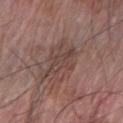<tbp_lesion>
  <biopsy_status>not biopsied; imaged during a skin examination</biopsy_status>
  <image>
    <source>total-body photography crop</source>
    <field_of_view_mm>15</field_of_view_mm>
  </image>
  <lesion_size>
    <long_diameter_mm_approx>3.5</long_diameter_mm_approx>
  </lesion_size>
  <lighting>white-light</lighting>
  <site>right forearm</site>
  <patient>
    <sex>male</sex>
    <age_approx>65</age_approx>
  </patient>
  <automated_metrics>
    <area_mm2_approx>7.0</area_mm2_approx>
    <eccentricity>0.6</eccentricity>
    <shape_asymmetry>0.2</shape_asymmetry>
  </automated_metrics>
</tbp_lesion>